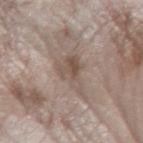<case>
  <biopsy_status>not biopsied; imaged during a skin examination</biopsy_status>
  <image>
    <source>total-body photography crop</source>
    <field_of_view_mm>15</field_of_view_mm>
  </image>
  <automated_metrics>
    <area_mm2_approx>9.5</area_mm2_approx>
    <eccentricity>0.85</eccentricity>
    <shape_asymmetry>0.5</shape_asymmetry>
    <border_irregularity_0_10>6.0</border_irregularity_0_10>
    <color_variation_0_10>6.0</color_variation_0_10>
    <peripheral_color_asymmetry>2.5</peripheral_color_asymmetry>
    <nevus_likeness_0_100>0</nevus_likeness_0_100>
  </automated_metrics>
  <lighting>white-light</lighting>
  <lesion_size>
    <long_diameter_mm_approx>5.5</long_diameter_mm_approx>
  </lesion_size>
  <site>left forearm</site>
  <patient>
    <sex>female</sex>
    <age_approx>75</age_approx>
  </patient>
</case>